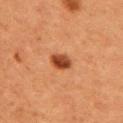Captured during whole-body skin photography for melanoma surveillance; the lesion was not biopsied. From the left upper arm. A region of skin cropped from a whole-body photographic capture, roughly 15 mm wide. Imaged with cross-polarized lighting. The lesion-visualizer software estimated an average lesion color of about L≈38 a*≈26 b*≈33 (CIELAB) and a normalized border contrast of about 11. Approximately 2.5 mm at its widest. A female subject, aged 38–42.